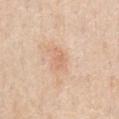Assessment:
Part of a total-body skin-imaging series; this lesion was reviewed on a skin check and was not flagged for biopsy.
Background:
A 15 mm close-up tile from a total-body photography series done for melanoma screening. Imaged with white-light lighting. A male patient, aged 58 to 62. Approximately 2.5 mm at its widest. The lesion is on the chest.